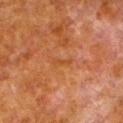Clinical impression: The lesion was tiled from a total-body skin photograph and was not biopsied. Background: Located on the leg. Cropped from a total-body skin-imaging series; the visible field is about 15 mm. The patient is a male in their 80s.